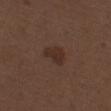Assessment: Recorded during total-body skin imaging; not selected for excision or biopsy. Image and clinical context: The lesion is located on the leg. Imaged with white-light lighting. The patient is a male in their 70s. The lesion's longest dimension is about 3 mm. Cropped from a whole-body photographic skin survey; the tile spans about 15 mm.